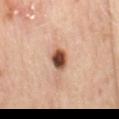Case summary:
- biopsy status — no biopsy performed (imaged during a skin exam)
- lighting — cross-polarized illumination
- location — the mid back
- image-analysis metrics — an average lesion color of about L≈50 a*≈23 b*≈31 (CIELAB), about 21 CIELAB-L* units darker than the surrounding skin, and a lesion-to-skin contrast of about 13 (normalized; higher = more distinct); an automated nevus-likeness rating near 100 out of 100 and a detector confidence of about 100 out of 100 that the crop contains a lesion
- acquisition — ~15 mm crop, total-body skin-cancer survey
- diameter — ~3 mm (longest diameter)
- patient — female, about 60 years old